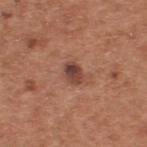biopsy_status: not biopsied; imaged during a skin examination
automated_metrics:
  shape_asymmetry: 0.2
  color_variation_0_10: 6.5
  peripheral_color_asymmetry: 2.0
  nevus_likeness_0_100: 30
patient:
  sex: male
  age_approx: 65
image:
  source: total-body photography crop
  field_of_view_mm: 15
lighting: white-light
site: upper back